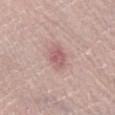This lesion was catalogued during total-body skin photography and was not selected for biopsy. Cropped from a total-body skin-imaging series; the visible field is about 15 mm. Automated tile analysis of the lesion measured an average lesion color of about L≈58 a*≈23 b*≈20 (CIELAB) and a lesion-to-skin contrast of about 6.5 (normalized; higher = more distinct). It also reported a border-irregularity rating of about 2/10, internal color variation of about 3 on a 0–10 scale, and radial color variation of about 1. The recorded lesion diameter is about 3 mm. A male subject aged 33–37. The lesion is on the right lower leg.